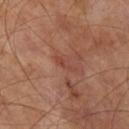{
  "lighting": "cross-polarized",
  "patient": {
    "sex": "male",
    "age_approx": 70
  },
  "lesion_size": {
    "long_diameter_mm_approx": 2.5
  },
  "image": {
    "source": "total-body photography crop",
    "field_of_view_mm": 15
  },
  "site": "right lower leg"
}